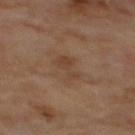Assessment: Captured during whole-body skin photography for melanoma surveillance; the lesion was not biopsied. Clinical summary: A male subject approximately 70 years of age. A 15 mm close-up extracted from a 3D total-body photography capture. The lesion is on the mid back. Approximately 4 mm at its widest. This is a cross-polarized tile. Automated tile analysis of the lesion measured a lesion area of about 6 mm² and a shape eccentricity near 0.85. The analysis additionally found radial color variation of about 0.5.